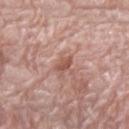workup = no biopsy performed (imaged during a skin exam); image source = total-body-photography crop, ~15 mm field of view; subject = male, aged 78–82; anatomic site = the right forearm.